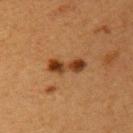Assessment: No biopsy was performed on this lesion — it was imaged during a full skin examination and was not determined to be concerning. Background: A lesion tile, about 15 mm wide, cut from a 3D total-body photograph. The lesion's longest dimension is about 4.5 mm. From the upper back. Imaged with cross-polarized lighting. The patient is a female roughly 40 years of age.